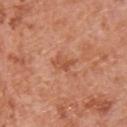Q: Was this lesion biopsied?
A: imaged on a skin check; not biopsied
Q: Lesion size?
A: about 2.5 mm
Q: Where on the body is the lesion?
A: the upper back
Q: How was the tile lit?
A: white-light
Q: What kind of image is this?
A: total-body-photography crop, ~15 mm field of view
Q: What are the patient's age and sex?
A: male, aged approximately 65
Q: What did automated image analysis measure?
A: an area of roughly 3 mm² and an outline eccentricity of about 0.8 (0 = round, 1 = elongated); an average lesion color of about L≈53 a*≈27 b*≈35 (CIELAB) and roughly 8 lightness units darker than nearby skin; a border-irregularity rating of about 4/10, a within-lesion color-variation index near 3/10, and peripheral color asymmetry of about 1